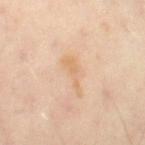notes: total-body-photography surveillance lesion; no biopsy
body site: the left leg
diameter: about 4 mm
automated lesion analysis: an average lesion color of about L≈69 a*≈18 b*≈36 (CIELAB), a lesion–skin lightness drop of about 6, and a normalized border contrast of about 5.5; border irregularity of about 7.5 on a 0–10 scale, a color-variation rating of about 0.5/10, and radial color variation of about 0; a classifier nevus-likeness of about 0/100 and lesion-presence confidence of about 100/100
patient: male, aged around 50
imaging modality: total-body-photography crop, ~15 mm field of view